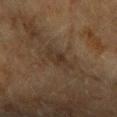patient: female, about 60 years old
location: the arm
image: ~15 mm crop, total-body skin-cancer survey